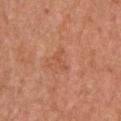notes: total-body-photography surveillance lesion; no biopsy | image source: ~15 mm tile from a whole-body skin photo | automated lesion analysis: an automated nevus-likeness rating near 0 out of 100 and a detector confidence of about 100 out of 100 that the crop contains a lesion | illumination: white-light illumination | location: the arm | subject: male, in their mid- to late 60s | diameter: ≈2.5 mm.